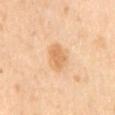Impression:
Captured during whole-body skin photography for melanoma surveillance; the lesion was not biopsied.
Acquisition and patient details:
The subject is a female about 55 years old. A region of skin cropped from a whole-body photographic capture, roughly 15 mm wide. Located on the mid back.